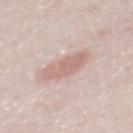{
  "biopsy_status": "not biopsied; imaged during a skin examination",
  "patient": {
    "sex": "male",
    "age_approx": 25
  },
  "image": {
    "source": "total-body photography crop",
    "field_of_view_mm": 15
  },
  "lesion_size": {
    "long_diameter_mm_approx": 4.5
  },
  "automated_metrics": {
    "cielab_L": 65,
    "cielab_a": 19,
    "cielab_b": 23,
    "vs_skin_darker_L": 10.0,
    "vs_skin_contrast_norm": 6.0
  },
  "site": "mid back"
}